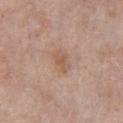Assessment:
Imaged during a routine full-body skin examination; the lesion was not biopsied and no histopathology is available.
Acquisition and patient details:
The patient is a male roughly 65 years of age. A 15 mm close-up extracted from a 3D total-body photography capture. The lesion is on the chest. The lesion's longest dimension is about 3 mm.